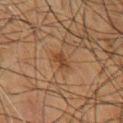The lesion was tiled from a total-body skin photograph and was not biopsied. From the chest. Cropped from a total-body skin-imaging series; the visible field is about 15 mm. The subject is a male approximately 65 years of age. Imaged with cross-polarized lighting.